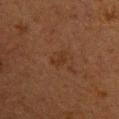Impression:
Captured during whole-body skin photography for melanoma surveillance; the lesion was not biopsied.
Context:
A 15 mm close-up extracted from a 3D total-body photography capture. The lesion is on the upper back. The subject is a female approximately 40 years of age.